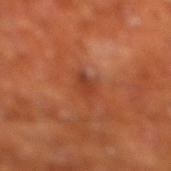Part of a total-body skin-imaging series; this lesion was reviewed on a skin check and was not flagged for biopsy. A male subject aged 63–67. The lesion is located on the right lower leg. Longest diameter approximately 2.5 mm. A close-up tile cropped from a whole-body skin photograph, about 15 mm across.